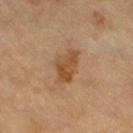The lesion is located on the left leg.
The lesion-visualizer software estimated an area of roughly 8 mm² and an eccentricity of roughly 0.85. The software also gave a mean CIELAB color near L≈47 a*≈19 b*≈35, a lesion–skin lightness drop of about 9, and a normalized lesion–skin contrast near 7.5. And it measured a border-irregularity index near 2.5/10, a color-variation rating of about 3.5/10, and peripheral color asymmetry of about 1. And it measured a nevus-likeness score of about 20/100 and lesion-presence confidence of about 100/100.
Measured at roughly 4 mm in maximum diameter.
Imaged with cross-polarized lighting.
Cropped from a whole-body photographic skin survey; the tile spans about 15 mm.
A male patient aged 63 to 67.